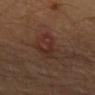Imaged during a routine full-body skin examination; the lesion was not biopsied and no histopathology is available.
A roughly 15 mm field-of-view crop from a total-body skin photograph.
From the left forearm.
A male patient, aged around 85 or older.